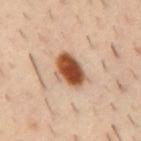Located on the mid back.
A 15 mm crop from a total-body photograph taken for skin-cancer surveillance.
Measured at roughly 4.5 mm in maximum diameter.
A male patient, aged approximately 40.